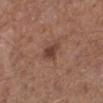| feature | finding |
|---|---|
| follow-up | total-body-photography surveillance lesion; no biopsy |
| tile lighting | white-light illumination |
| location | the left lower leg |
| diameter | ≈3 mm |
| patient | male, aged approximately 60 |
| image source | total-body-photography crop, ~15 mm field of view |